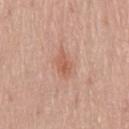Captured during whole-body skin photography for melanoma surveillance; the lesion was not biopsied.
Longest diameter approximately 3 mm.
A male subject aged 53 to 57.
The lesion is located on the lower back.
This image is a 15 mm lesion crop taken from a total-body photograph.
Captured under white-light illumination.